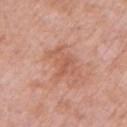Captured during whole-body skin photography for melanoma surveillance; the lesion was not biopsied. On the left upper arm. A roughly 15 mm field-of-view crop from a total-body skin photograph. A female patient aged 68 to 72. The lesion's longest dimension is about 4 mm. Automated image analysis of the tile measured a nevus-likeness score of about 0/100 and a lesion-detection confidence of about 100/100.